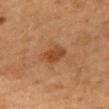The lesion was photographed on a routine skin check and not biopsied; there is no pathology result.
The patient is a female aged 58 to 62.
The lesion-visualizer software estimated a mean CIELAB color near L≈40 a*≈21 b*≈34. And it measured a border-irregularity rating of about 2/10, a color-variation rating of about 2/10, and peripheral color asymmetry of about 0.5. The analysis additionally found a classifier nevus-likeness of about 65/100 and lesion-presence confidence of about 100/100.
Approximately 3 mm at its widest.
Located on the mid back.
A roughly 15 mm field-of-view crop from a total-body skin photograph.